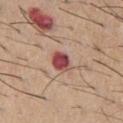Q: Was a biopsy performed?
A: total-body-photography surveillance lesion; no biopsy
Q: How large is the lesion?
A: ~3 mm (longest diameter)
Q: Where on the body is the lesion?
A: the chest
Q: What lighting was used for the tile?
A: white-light illumination
Q: What kind of image is this?
A: total-body-photography crop, ~15 mm field of view
Q: Who is the patient?
A: male, aged 58–62
Q: What did automated image analysis measure?
A: a lesion area of about 5 mm², an outline eccentricity of about 0.6 (0 = round, 1 = elongated), and a symmetry-axis asymmetry near 0.15; border irregularity of about 1 on a 0–10 scale, a within-lesion color-variation index near 3.5/10, and peripheral color asymmetry of about 1